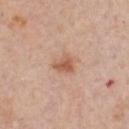Findings:
* subject: male, aged around 60
* lighting: white-light illumination
* body site: the chest
* automated lesion analysis: an area of roughly 4 mm², an outline eccentricity of about 0.6 (0 = round, 1 = elongated), and a shape-asymmetry score of about 0.35 (0 = symmetric)
* image source: 15 mm crop, total-body photography